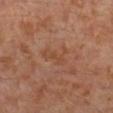The lesion was photographed on a routine skin check and not biopsied; there is no pathology result.
The lesion is located on the left leg.
A roughly 15 mm field-of-view crop from a total-body skin photograph.
The subject is a male aged 28 to 32.
Measured at roughly 4 mm in maximum diameter.